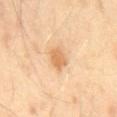Captured during whole-body skin photography for melanoma surveillance; the lesion was not biopsied. Cropped from a total-body skin-imaging series; the visible field is about 15 mm. The patient is a male aged 38 to 42. Captured under cross-polarized illumination. The total-body-photography lesion software estimated a mean CIELAB color near L≈69 a*≈22 b*≈42, a lesion–skin lightness drop of about 11, and a lesion-to-skin contrast of about 7 (normalized; higher = more distinct). The analysis additionally found a color-variation rating of about 2/10 and peripheral color asymmetry of about 0.5. The software also gave a detector confidence of about 100 out of 100 that the crop contains a lesion. Approximately 2.5 mm at its widest. The lesion is on the mid back.